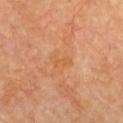Q: Was this lesion biopsied?
A: imaged on a skin check; not biopsied
Q: Lesion location?
A: the chest
Q: What kind of image is this?
A: 15 mm crop, total-body photography
Q: Patient demographics?
A: female, aged around 60